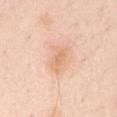Clinical summary:
A roughly 15 mm field-of-view crop from a total-body skin photograph. Captured under white-light illumination. Approximately 4 mm at its widest. A female subject, aged approximately 70. From the chest.